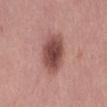Impression: Imaged during a routine full-body skin examination; the lesion was not biopsied and no histopathology is available. Context: A lesion tile, about 15 mm wide, cut from a 3D total-body photograph. The lesion is on the right thigh. A female patient, aged around 40.